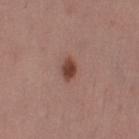<case>
  <patient>
    <sex>female</sex>
    <age_approx>50</age_approx>
  </patient>
  <image>
    <source>total-body photography crop</source>
    <field_of_view_mm>15</field_of_view_mm>
  </image>
  <site>left thigh</site>
  <lighting>white-light</lighting>
</case>